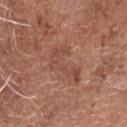{
  "biopsy_status": "not biopsied; imaged during a skin examination",
  "image": {
    "source": "total-body photography crop",
    "field_of_view_mm": 15
  },
  "site": "head or neck",
  "automated_metrics": {
    "area_mm2_approx": 8.5,
    "eccentricity": 0.9,
    "border_irregularity_0_10": 7.5,
    "peripheral_color_asymmetry": 0.5,
    "nevus_likeness_0_100": 0,
    "lesion_detection_confidence_0_100": 100
  },
  "patient": {
    "sex": "male",
    "age_approx": 75
  },
  "lesion_size": {
    "long_diameter_mm_approx": 5.5
  },
  "lighting": "white-light"
}